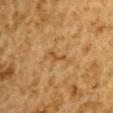  biopsy_status: not biopsied; imaged during a skin examination
  site: right upper arm
  image:
    source: total-body photography crop
    field_of_view_mm: 15
  patient:
    sex: male
    age_approx: 85+